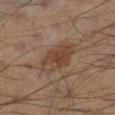Background:
A male patient, aged 53–57. Automated tile analysis of the lesion measured an average lesion color of about L≈39 a*≈16 b*≈26 (CIELAB), a lesion–skin lightness drop of about 8, and a lesion-to-skin contrast of about 7.5 (normalized; higher = more distinct). The lesion is located on the right lower leg. A roughly 15 mm field-of-view crop from a total-body skin photograph. The recorded lesion diameter is about 5 mm. Captured under cross-polarized illumination.